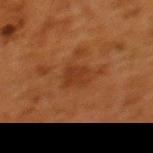{
  "biopsy_status": "not biopsied; imaged during a skin examination",
  "site": "back",
  "patient": {
    "sex": "female",
    "age_approx": 50
  },
  "image": {
    "source": "total-body photography crop",
    "field_of_view_mm": 15
  }
}